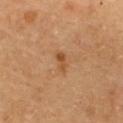Impression: No biopsy was performed on this lesion — it was imaged during a full skin examination and was not determined to be concerning. Clinical summary: From the upper back. A female patient in their 60s. A 15 mm close-up tile from a total-body photography series done for melanoma screening. Longest diameter approximately 3 mm. Captured under cross-polarized illumination.